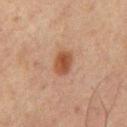Background: From the upper back. The tile uses cross-polarized illumination. Automated image analysis of the tile measured a footprint of about 5.5 mm², a shape eccentricity near 0.7, and two-axis asymmetry of about 0.15. And it measured a lesion color around L≈39 a*≈20 b*≈28 in CIELAB and a lesion-to-skin contrast of about 9 (normalized; higher = more distinct). And it measured an automated nevus-likeness rating near 100 out of 100 and lesion-presence confidence of about 100/100. A male subject aged around 65. Cropped from a total-body skin-imaging series; the visible field is about 15 mm.